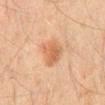<record>
  <biopsy_status>not biopsied; imaged during a skin examination</biopsy_status>
  <lesion_size>
    <long_diameter_mm_approx>3.5</long_diameter_mm_approx>
  </lesion_size>
  <site>mid back</site>
  <patient>
    <sex>male</sex>
    <age_approx>45</age_approx>
  </patient>
  <automated_metrics>
    <cielab_L>50</cielab_L>
    <cielab_a>20</cielab_a>
    <cielab_b>32</cielab_b>
    <vs_skin_darker_L>9.0</vs_skin_darker_L>
    <vs_skin_contrast_norm>7.0</vs_skin_contrast_norm>
    <lesion_detection_confidence_0_100>100</lesion_detection_confidence_0_100>
  </automated_metrics>
  <image>
    <source>total-body photography crop</source>
    <field_of_view_mm>15</field_of_view_mm>
  </image>
  <lighting>cross-polarized</lighting>
</record>